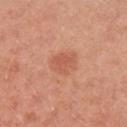Assessment: Captured during whole-body skin photography for melanoma surveillance; the lesion was not biopsied. Context: From the arm. About 3.5 mm across. Cropped from a whole-body photographic skin survey; the tile spans about 15 mm. Imaged with white-light lighting. A male patient approximately 30 years of age.